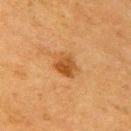Imaged during a routine full-body skin examination; the lesion was not biopsied and no histopathology is available.
A close-up tile cropped from a whole-body skin photograph, about 15 mm across.
Approximately 3 mm at its widest.
A female patient aged 53 to 57.
This is a cross-polarized tile.
The lesion is located on the arm.